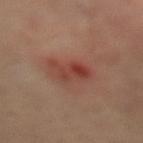Case summary:
* biopsy status: catalogued during a skin exam; not biopsied
* imaging modality: total-body-photography crop, ~15 mm field of view
* tile lighting: cross-polarized illumination
* image-analysis metrics: a footprint of about 7 mm², an outline eccentricity of about 0.8 (0 = round, 1 = elongated), and a shape-asymmetry score of about 0.3 (0 = symmetric); a classifier nevus-likeness of about 0/100
* anatomic site: the right leg
* patient: female, aged around 40
* lesion diameter: ~4 mm (longest diameter)